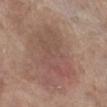Impression: Part of a total-body skin-imaging series; this lesion was reviewed on a skin check and was not flagged for biopsy. Clinical summary: The lesion's longest dimension is about 7 mm. Imaged with white-light lighting. Located on the right lower leg. A 15 mm close-up extracted from a 3D total-body photography capture. A female subject approximately 85 years of age or older.